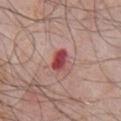Findings:
- follow-up · imaged on a skin check; not biopsied
- subject · male, aged 78–82
- site · the front of the torso
- size · ≈2.5 mm
- illumination · white-light illumination
- imaging modality · 15 mm crop, total-body photography